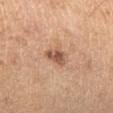Recorded during total-body skin imaging; not selected for excision or biopsy.
The lesion's longest dimension is about 3 mm.
Cropped from a whole-body photographic skin survey; the tile spans about 15 mm.
A male patient aged around 60.
Captured under cross-polarized illumination.
On the right lower leg.